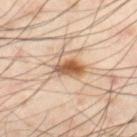Case summary:
- follow-up · no biopsy performed (imaged during a skin exam)
- patient · male, roughly 55 years of age
- automated metrics · a mean CIELAB color near L≈60 a*≈20 b*≈34, about 15 CIELAB-L* units darker than the surrounding skin, and a normalized border contrast of about 9.5; a classifier nevus-likeness of about 95/100 and a detector confidence of about 100 out of 100 that the crop contains a lesion
- imaging modality · total-body-photography crop, ~15 mm field of view
- lesion diameter · ~3.5 mm (longest diameter)
- anatomic site · the left thigh